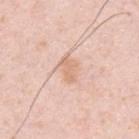No biopsy was performed on this lesion — it was imaged during a full skin examination and was not determined to be concerning.
A 15 mm close-up extracted from a 3D total-body photography capture.
From the back.
The recorded lesion diameter is about 3 mm.
A male subject, roughly 35 years of age.
The tile uses white-light illumination.
An algorithmic analysis of the crop reported a lesion color around L≈70 a*≈20 b*≈31 in CIELAB, roughly 8 lightness units darker than nearby skin, and a normalized border contrast of about 5.5. And it measured border irregularity of about 3 on a 0–10 scale, a color-variation rating of about 1.5/10, and radial color variation of about 0.5.